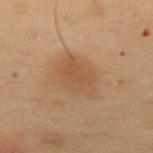Notes:
• workup — no biopsy performed (imaged during a skin exam)
• size — about 5 mm
• image — ~15 mm tile from a whole-body skin photo
• subject — male, aged around 55
• TBP lesion metrics — a lesion area of about 15 mm² and an outline eccentricity of about 0.7 (0 = round, 1 = elongated); an average lesion color of about L≈42 a*≈16 b*≈29 (CIELAB), about 6 CIELAB-L* units darker than the surrounding skin, and a normalized border contrast of about 5.5; a border-irregularity index near 2.5/10 and radial color variation of about 1
• site — the mid back